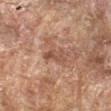This lesion was catalogued during total-body skin photography and was not selected for biopsy. A male subject, roughly 60 years of age. The lesion is located on the left forearm. Imaged with cross-polarized lighting. Measured at roughly 2.5 mm in maximum diameter. This image is a 15 mm lesion crop taken from a total-body photograph.